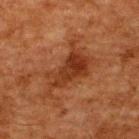The lesion was tiled from a total-body skin photograph and was not biopsied. The lesion is located on the upper back. Approximately 7 mm at its widest. A region of skin cropped from a whole-body photographic capture, roughly 15 mm wide. A male patient, roughly 60 years of age.